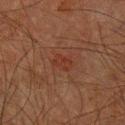Notes:
• site: the left forearm
• imaging modality: ~15 mm tile from a whole-body skin photo
• tile lighting: cross-polarized illumination
• subject: male, aged 58–62